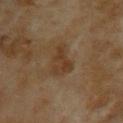This is a cross-polarized tile.
The lesion is located on the upper back.
The total-body-photography lesion software estimated a border-irregularity index near 5/10 and a color-variation rating of about 2.5/10.
A female patient, aged 58 to 62.
A close-up tile cropped from a whole-body skin photograph, about 15 mm across.